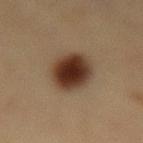The lesion was tiled from a total-body skin photograph and was not biopsied.
A 15 mm close-up extracted from a 3D total-body photography capture.
Located on the lower back.
This is a cross-polarized tile.
The subject is a female about 40 years old.